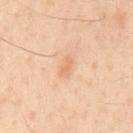The lesion was photographed on a routine skin check and not biopsied; there is no pathology result.
Located on the mid back.
Imaged with cross-polarized lighting.
An algorithmic analysis of the crop reported an area of roughly 3 mm², an eccentricity of roughly 0.9, and a symmetry-axis asymmetry near 0.35. It also reported border irregularity of about 3 on a 0–10 scale and a within-lesion color-variation index near 0.5/10. It also reported a classifier nevus-likeness of about 0/100 and a detector confidence of about 100 out of 100 that the crop contains a lesion.
A 15 mm close-up extracted from a 3D total-body photography capture.
About 3 mm across.
A male patient, in their mid-50s.